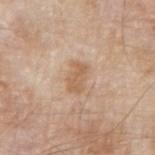A 15 mm crop from a total-body photograph taken for skin-cancer surveillance. This is a white-light tile. A male subject, roughly 80 years of age. The lesion is on the right upper arm.